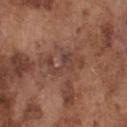notes — catalogued during a skin exam; not biopsied
patient — male, aged 73 to 77
image — total-body-photography crop, ~15 mm field of view
body site — the chest
automated lesion analysis — an average lesion color of about L≈42 a*≈20 b*≈25 (CIELAB), roughly 7 lightness units darker than nearby skin, and a normalized border contrast of about 6.5
tile lighting — white-light illumination